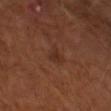Case summary:
- workup · imaged on a skin check; not biopsied
- TBP lesion metrics · a shape-asymmetry score of about 0.45 (0 = symmetric); an average lesion color of about L≈28 a*≈20 b*≈26 (CIELAB), roughly 5 lightness units darker than nearby skin, and a normalized border contrast of about 5.5; an automated nevus-likeness rating near 5 out of 100 and a detector confidence of about 100 out of 100 that the crop contains a lesion
- diameter · ~2.5 mm (longest diameter)
- site · the right upper arm
- subject · male, aged approximately 65
- image · ~15 mm tile from a whole-body skin photo